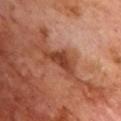workup — catalogued during a skin exam; not biopsied
patient — male, roughly 70 years of age
location — the chest
automated lesion analysis — a lesion area of about 5.5 mm²; a lesion color around L≈40 a*≈27 b*≈32 in CIELAB, a lesion–skin lightness drop of about 12, and a lesion-to-skin contrast of about 9.5 (normalized; higher = more distinct); border irregularity of about 6 on a 0–10 scale, a within-lesion color-variation index near 1.5/10, and radial color variation of about 0.5; a classifier nevus-likeness of about 5/100 and a lesion-detection confidence of about 100/100
imaging modality — ~15 mm crop, total-body skin-cancer survey
lighting — cross-polarized illumination